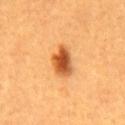The lesion was tiled from a total-body skin photograph and was not biopsied.